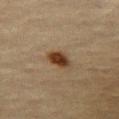Clinical impression:
The lesion was tiled from a total-body skin photograph and was not biopsied.
Image and clinical context:
A female subject, in their mid- to late 60s. The lesion is located on the left thigh. This is a cross-polarized tile. Cropped from a total-body skin-imaging series; the visible field is about 15 mm.